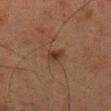This lesion was catalogued during total-body skin photography and was not selected for biopsy.
Imaged with cross-polarized lighting.
A region of skin cropped from a whole-body photographic capture, roughly 15 mm wide.
The patient is a male roughly 60 years of age.
Measured at roughly 2.5 mm in maximum diameter.
Located on the back.